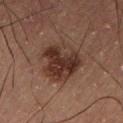A 15 mm close-up tile from a total-body photography series done for melanoma screening. Located on the abdomen. The patient is a male aged around 55. The lesion's longest dimension is about 5.5 mm. This is a cross-polarized tile.